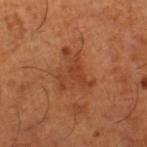Part of a total-body skin-imaging series; this lesion was reviewed on a skin check and was not flagged for biopsy.
An algorithmic analysis of the crop reported a footprint of about 12 mm², an outline eccentricity of about 0.7 (0 = round, 1 = elongated), and a symmetry-axis asymmetry near 0.6. And it measured an average lesion color of about L≈43 a*≈27 b*≈36 (CIELAB), a lesion–skin lightness drop of about 8, and a normalized border contrast of about 6. And it measured border irregularity of about 7.5 on a 0–10 scale, a color-variation rating of about 3/10, and peripheral color asymmetry of about 1.
Cropped from a total-body skin-imaging series; the visible field is about 15 mm.
On the leg.
The patient is a male approximately 65 years of age.
Captured under cross-polarized illumination.
Longest diameter approximately 5.5 mm.